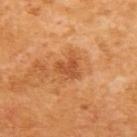The lesion was tiled from a total-body skin photograph and was not biopsied.
Captured under cross-polarized illumination.
The subject is a female aged 53–57.
From the upper back.
A roughly 15 mm field-of-view crop from a total-body skin photograph.